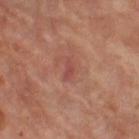Notes:
• biopsy status — no biopsy performed (imaged during a skin exam)
• anatomic site — the left leg
• lesion diameter — ≈2.5 mm
• automated lesion analysis — roughly 7 lightness units darker than nearby skin and a normalized lesion–skin contrast near 6; a border-irregularity rating of about 3.5/10, a within-lesion color-variation index near 0/10, and radial color variation of about 0; a classifier nevus-likeness of about 0/100 and a detector confidence of about 100 out of 100 that the crop contains a lesion
• subject — female, in their mid- to late 60s
• acquisition — ~15 mm crop, total-body skin-cancer survey
• tile lighting — cross-polarized illumination